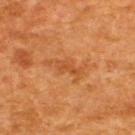Imaged during a routine full-body skin examination; the lesion was not biopsied and no histopathology is available.
A male subject in their 60s.
About 3 mm across.
Automated tile analysis of the lesion measured a lesion color around L≈47 a*≈27 b*≈41 in CIELAB and a lesion-to-skin contrast of about 5 (normalized; higher = more distinct). The analysis additionally found a border-irregularity rating of about 4/10 and radial color variation of about 0.5. It also reported a classifier nevus-likeness of about 0/100 and lesion-presence confidence of about 100/100.
Cropped from a whole-body photographic skin survey; the tile spans about 15 mm.
The lesion is on the upper back.